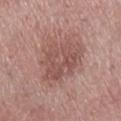A 15 mm close-up extracted from a 3D total-body photography capture. Located on the leg. A female subject aged 68 to 72. This is a white-light tile.